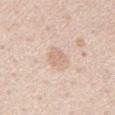The lesion was tiled from a total-body skin photograph and was not biopsied.
A close-up tile cropped from a whole-body skin photograph, about 15 mm across.
A male patient in their 60s.
The tile uses white-light illumination.
Located on the chest.